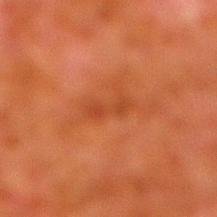Captured during whole-body skin photography for melanoma surveillance; the lesion was not biopsied. A roughly 15 mm field-of-view crop from a total-body skin photograph. A male patient, in their 80s. On the leg.